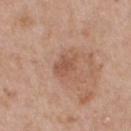This lesion was catalogued during total-body skin photography and was not selected for biopsy. A 15 mm close-up extracted from a 3D total-body photography capture. From the upper back. A male subject, roughly 55 years of age.